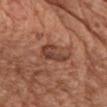Findings:
- workup: imaged on a skin check; not biopsied
- TBP lesion metrics: an area of roughly 8 mm², an outline eccentricity of about 0.85 (0 = round, 1 = elongated), and two-axis asymmetry of about 0.35; a lesion–skin lightness drop of about 10 and a normalized border contrast of about 7.5
- lighting: white-light illumination
- body site: the chest
- subject: female, approximately 75 years of age
- image source: ~15 mm crop, total-body skin-cancer survey
- lesion diameter: about 4.5 mm